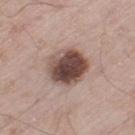Case summary:
– biopsy status: catalogued during a skin exam; not biopsied
– lighting: white-light
– patient: male, aged around 60
– imaging modality: 15 mm crop, total-body photography
– anatomic site: the right thigh